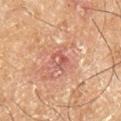{"biopsy_status": "not biopsied; imaged during a skin examination", "patient": {"sex": "male", "age_approx": 65}, "site": "right lower leg", "lesion_size": {"long_diameter_mm_approx": 2.5}, "image": {"source": "total-body photography crop", "field_of_view_mm": 15}, "automated_metrics": {"cielab_L": 45, "cielab_a": 23, "cielab_b": 24, "vs_skin_contrast_norm": 5.5, "peripheral_color_asymmetry": 2.5}, "lighting": "cross-polarized"}